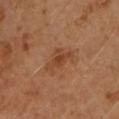Assessment: Part of a total-body skin-imaging series; this lesion was reviewed on a skin check and was not flagged for biopsy. Context: Longest diameter approximately 4 mm. The lesion-visualizer software estimated an area of roughly 7 mm². The analysis additionally found border irregularity of about 5 on a 0–10 scale and internal color variation of about 3 on a 0–10 scale. It also reported a nevus-likeness score of about 10/100 and a detector confidence of about 100 out of 100 that the crop contains a lesion. This is a cross-polarized tile. The subject is a male aged 43 to 47. On the left upper arm. A 15 mm crop from a total-body photograph taken for skin-cancer surveillance.